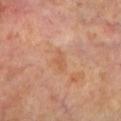Clinical impression: Part of a total-body skin-imaging series; this lesion was reviewed on a skin check and was not flagged for biopsy. Image and clinical context: A male patient aged approximately 70. The lesion is located on the left lower leg. Imaged with cross-polarized lighting. The lesion's longest dimension is about 2.5 mm. A 15 mm crop from a total-body photograph taken for skin-cancer surveillance.